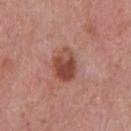Clinical impression:
The lesion was tiled from a total-body skin photograph and was not biopsied.
Image and clinical context:
An algorithmic analysis of the crop reported a lesion area of about 9.5 mm² and an eccentricity of roughly 0.7. The analysis additionally found a mean CIELAB color near L≈47 a*≈25 b*≈28, roughly 12 lightness units darker than nearby skin, and a normalized lesion–skin contrast near 9. And it measured a border-irregularity index near 2/10 and internal color variation of about 7.5 on a 0–10 scale. The recorded lesion diameter is about 4 mm. Cropped from a whole-body photographic skin survey; the tile spans about 15 mm. Located on the chest. A male subject approximately 50 years of age. The tile uses white-light illumination.